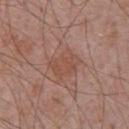The lesion was photographed on a routine skin check and not biopsied; there is no pathology result. The lesion-visualizer software estimated an area of roughly 8 mm², an eccentricity of roughly 0.7, and a shape-asymmetry score of about 0.25 (0 = symmetric). It also reported border irregularity of about 2.5 on a 0–10 scale, a color-variation rating of about 2/10, and a peripheral color-asymmetry measure near 0.5. A 15 mm close-up tile from a total-body photography series done for melanoma screening. The lesion is located on the chest. A male subject, roughly 70 years of age. About 3.5 mm across.